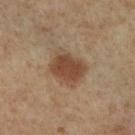Q: Is there a histopathology result?
A: total-body-photography surveillance lesion; no biopsy
Q: Who is the patient?
A: female, approximately 65 years of age
Q: Lesion size?
A: about 4.5 mm
Q: Illumination type?
A: cross-polarized illumination
Q: Lesion location?
A: the left leg
Q: What kind of image is this?
A: 15 mm crop, total-body photography
Q: What did automated image analysis measure?
A: a mean CIELAB color near L≈44 a*≈17 b*≈29; internal color variation of about 3.5 on a 0–10 scale